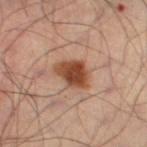The lesion was photographed on a routine skin check and not biopsied; there is no pathology result. From the left leg. A region of skin cropped from a whole-body photographic capture, roughly 15 mm wide. Imaged with cross-polarized lighting. Measured at roughly 4 mm in maximum diameter. The patient is a male aged 48 to 52. The lesion-visualizer software estimated a lesion area of about 8.5 mm², a shape eccentricity near 0.6, and a shape-asymmetry score of about 0.25 (0 = symmetric). And it measured a mean CIELAB color near L≈45 a*≈23 b*≈32 and a lesion–skin lightness drop of about 15. And it measured border irregularity of about 2.5 on a 0–10 scale, internal color variation of about 4 on a 0–10 scale, and a peripheral color-asymmetry measure near 1. And it measured an automated nevus-likeness rating near 100 out of 100.